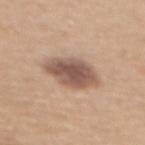No biopsy was performed on this lesion — it was imaged during a full skin examination and was not determined to be concerning. The tile uses white-light illumination. A region of skin cropped from a whole-body photographic capture, roughly 15 mm wide. The total-body-photography lesion software estimated an area of roughly 13 mm², a shape eccentricity near 0.7, and a symmetry-axis asymmetry near 0.15. And it measured a mean CIELAB color near L≈54 a*≈18 b*≈26, about 14 CIELAB-L* units darker than the surrounding skin, and a lesion-to-skin contrast of about 10 (normalized; higher = more distinct). The software also gave a color-variation rating of about 4/10 and radial color variation of about 1. Measured at roughly 5 mm in maximum diameter. The subject is a female aged 53–57. The lesion is on the upper back.